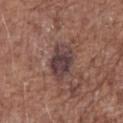The lesion was photographed on a routine skin check and not biopsied; there is no pathology result. This is a white-light tile. Cropped from a total-body skin-imaging series; the visible field is about 15 mm. The subject is a male aged around 70. The total-body-photography lesion software estimated an area of roughly 11 mm² and a shape-asymmetry score of about 0.2 (0 = symmetric). The software also gave border irregularity of about 2 on a 0–10 scale, a color-variation rating of about 4.5/10, and peripheral color asymmetry of about 1.5. On the abdomen.